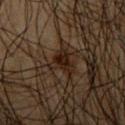notes: no biopsy performed (imaged during a skin exam)
lesion diameter: about 4 mm
site: the arm
patient: male, in their 50s
image source: total-body-photography crop, ~15 mm field of view
automated lesion analysis: a border-irregularity index near 5.5/10, a within-lesion color-variation index near 3/10, and radial color variation of about 1; a classifier nevus-likeness of about 0/100
lighting: cross-polarized